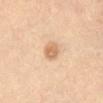Q: Was this lesion biopsied?
A: total-body-photography surveillance lesion; no biopsy
Q: What is the anatomic site?
A: the abdomen
Q: What is the lesion's diameter?
A: about 2.5 mm
Q: How was this image acquired?
A: total-body-photography crop, ~15 mm field of view
Q: What lighting was used for the tile?
A: cross-polarized
Q: What are the patient's age and sex?
A: female, in their mid-40s